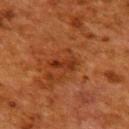This lesion was catalogued during total-body skin photography and was not selected for biopsy.
Imaged with cross-polarized lighting.
A close-up tile cropped from a whole-body skin photograph, about 15 mm across.
A female patient, roughly 50 years of age.
On the upper back.